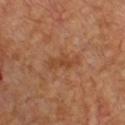biopsy_status: not biopsied; imaged during a skin examination
site: front of the torso
automated_metrics:
  area_mm2_approx: 5.0
  eccentricity: 0.9
  shape_asymmetry: 0.35
  color_variation_0_10: 2.0
  peripheral_color_asymmetry: 0.5
  nevus_likeness_0_100: 0
  lesion_detection_confidence_0_100: 100
image:
  source: total-body photography crop
  field_of_view_mm: 15
lesion_size:
  long_diameter_mm_approx: 4.0
patient:
  sex: male
  age_approx: 50
lighting: cross-polarized